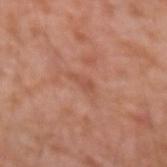Clinical impression:
The lesion was photographed on a routine skin check and not biopsied; there is no pathology result.
Context:
The lesion is on the left forearm. The tile uses white-light illumination. The subject is a male aged 73 to 77. The lesion's longest dimension is about 2.5 mm. A 15 mm crop from a total-body photograph taken for skin-cancer surveillance.